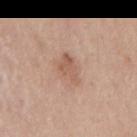Located on the mid back. The total-body-photography lesion software estimated a footprint of about 5.5 mm², an eccentricity of roughly 0.85, and a shape-asymmetry score of about 0.35 (0 = symmetric). The analysis additionally found a mean CIELAB color near L≈57 a*≈20 b*≈29 and a normalized lesion–skin contrast near 6. The analysis additionally found a border-irregularity index near 4/10 and a within-lesion color-variation index near 2/10. And it measured a classifier nevus-likeness of about 5/100 and a lesion-detection confidence of about 100/100. A male subject approximately 50 years of age. This is a white-light tile. A region of skin cropped from a whole-body photographic capture, roughly 15 mm wide. Longest diameter approximately 4 mm.